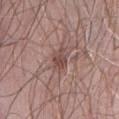This lesion was catalogued during total-body skin photography and was not selected for biopsy. Approximately 2.5 mm at its widest. The tile uses white-light illumination. From the abdomen. A 15 mm close-up extracted from a 3D total-body photography capture. A female patient aged approximately 65.